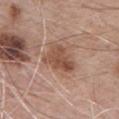No biopsy was performed on this lesion — it was imaged during a full skin examination and was not determined to be concerning.
Longest diameter approximately 4 mm.
Captured under white-light illumination.
Cropped from a total-body skin-imaging series; the visible field is about 15 mm.
Located on the chest.
A male subject, in their mid-60s.
The total-body-photography lesion software estimated an area of roughly 9 mm², an eccentricity of roughly 0.75, and a shape-asymmetry score of about 0.4 (0 = symmetric). The analysis additionally found a border-irregularity rating of about 4.5/10 and peripheral color asymmetry of about 1.5.